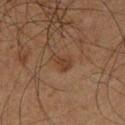The lesion was tiled from a total-body skin photograph and was not biopsied.
The lesion is located on the left lower leg.
The subject is a male roughly 65 years of age.
A 15 mm crop from a total-body photograph taken for skin-cancer surveillance.
Captured under cross-polarized illumination.
Approximately 2.5 mm at its widest.